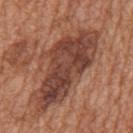{
  "biopsy_status": "not biopsied; imaged during a skin examination",
  "patient": {
    "sex": "male",
    "age_approx": 65
  },
  "site": "mid back",
  "image": {
    "source": "total-body photography crop",
    "field_of_view_mm": 15
  },
  "lighting": "white-light"
}